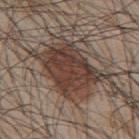Captured during whole-body skin photography for melanoma surveillance; the lesion was not biopsied. The subject is a male aged 43–47. About 8.5 mm across. A region of skin cropped from a whole-body photographic capture, roughly 15 mm wide. The lesion is on the mid back.